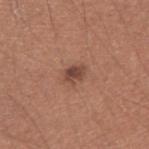biopsy_status: not biopsied; imaged during a skin examination
automated_metrics:
  area_mm2_approx: 4.0
  eccentricity: 0.65
  shape_asymmetry: 0.25
  border_irregularity_0_10: 3.0
  color_variation_0_10: 3.0
  peripheral_color_asymmetry: 1.0
  nevus_likeness_0_100: 60
  lesion_detection_confidence_0_100: 100
patient:
  sex: male
  age_approx: 35
site: right forearm
lesion_size:
  long_diameter_mm_approx: 2.5
image:
  source: total-body photography crop
  field_of_view_mm: 15
lighting: white-light